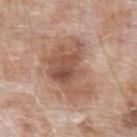Clinical impression: No biopsy was performed on this lesion — it was imaged during a full skin examination and was not determined to be concerning. Image and clinical context: The patient is a male aged 73 to 77. The lesion is located on the left forearm. A 15 mm close-up extracted from a 3D total-body photography capture.